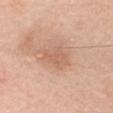Recorded during total-body skin imaging; not selected for excision or biopsy. A female patient approximately 50 years of age. A 15 mm close-up tile from a total-body photography series done for melanoma screening. On the chest.